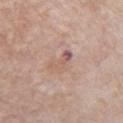Clinical impression:
The lesion was tiled from a total-body skin photograph and was not biopsied.
Context:
The lesion-visualizer software estimated border irregularity of about 5.5 on a 0–10 scale, a within-lesion color-variation index near 1/10, and peripheral color asymmetry of about 0. And it measured lesion-presence confidence of about 100/100. A male subject, in their mid-80s or older. The lesion's longest dimension is about 3.5 mm. The lesion is on the front of the torso. Cropped from a whole-body photographic skin survey; the tile spans about 15 mm. Captured under white-light illumination.